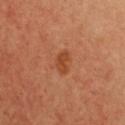Automated tile analysis of the lesion measured a lesion area of about 4 mm².
The lesion's longest dimension is about 2.5 mm.
A 15 mm close-up extracted from a 3D total-body photography capture.
This is a cross-polarized tile.
The patient is a female aged approximately 50.